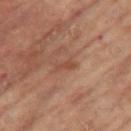Impression:
This lesion was catalogued during total-body skin photography and was not selected for biopsy.
Acquisition and patient details:
A female patient approximately 65 years of age. A lesion tile, about 15 mm wide, cut from a 3D total-body photograph. The lesion-visualizer software estimated an average lesion color of about L≈46 a*≈23 b*≈29 (CIELAB), roughly 7 lightness units darker than nearby skin, and a lesion-to-skin contrast of about 5.5 (normalized; higher = more distinct). And it measured a border-irregularity index near 4/10 and a within-lesion color-variation index near 0/10. It also reported a nevus-likeness score of about 0/100 and a lesion-detection confidence of about 100/100. The lesion is on the left leg. Approximately 2.5 mm at its widest.